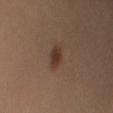<lesion>
  <biopsy_status>not biopsied; imaged during a skin examination</biopsy_status>
  <site>left upper arm</site>
  <patient>
    <sex>female</sex>
    <age_approx>40</age_approx>
  </patient>
  <image>
    <source>total-body photography crop</source>
    <field_of_view_mm>15</field_of_view_mm>
  </image>
  <automated_metrics>
    <area_mm2_approx>4.5</area_mm2_approx>
    <eccentricity>0.8</eccentricity>
    <shape_asymmetry>0.2</shape_asymmetry>
    <cielab_L>34</cielab_L>
    <cielab_a>17</cielab_a>
    <cielab_b>25</cielab_b>
    <vs_skin_darker_L>9.0</vs_skin_darker_L>
    <vs_skin_contrast_norm>8.5</vs_skin_contrast_norm>
    <lesion_detection_confidence_0_100>100</lesion_detection_confidence_0_100>
  </automated_metrics>
  <lighting>cross-polarized</lighting>
</lesion>